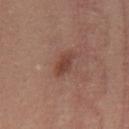Q: Was this lesion biopsied?
A: total-body-photography surveillance lesion; no biopsy